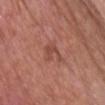Clinical impression: Recorded during total-body skin imaging; not selected for excision or biopsy. Background: The subject is a male aged approximately 65. A lesion tile, about 15 mm wide, cut from a 3D total-body photograph. Located on the chest.